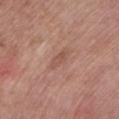Recorded during total-body skin imaging; not selected for excision or biopsy.
The lesion is on the front of the torso.
A female subject in their mid- to late 60s.
About 3 mm across.
Captured under white-light illumination.
A 15 mm close-up tile from a total-body photography series done for melanoma screening.
An algorithmic analysis of the crop reported a mean CIELAB color near L≈53 a*≈21 b*≈27, a lesion–skin lightness drop of about 7, and a normalized border contrast of about 5. The software also gave a detector confidence of about 100 out of 100 that the crop contains a lesion.